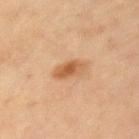  biopsy_status: not biopsied; imaged during a skin examination
  lesion_size:
    long_diameter_mm_approx: 3.5
  automated_metrics:
    area_mm2_approx: 5.0
    eccentricity: 0.8
    border_irregularity_0_10: 2.0
    color_variation_0_10: 3.5
    peripheral_color_asymmetry: 1.0
  patient:
    sex: female
    age_approx: 40
  image:
    source: total-body photography crop
    field_of_view_mm: 15
  site: left upper arm